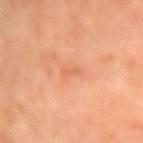Acquisition and patient details: Located on the right upper arm. A female subject, in their mid-60s. A roughly 15 mm field-of-view crop from a total-body skin photograph.